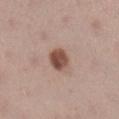Part of a total-body skin-imaging series; this lesion was reviewed on a skin check and was not flagged for biopsy. A close-up tile cropped from a whole-body skin photograph, about 15 mm across. The lesion-visualizer software estimated an average lesion color of about L≈49 a*≈20 b*≈25 (CIELAB), about 15 CIELAB-L* units darker than the surrounding skin, and a normalized lesion–skin contrast near 10.5. It also reported a color-variation rating of about 4/10 and peripheral color asymmetry of about 1.5. And it measured a nevus-likeness score of about 100/100 and lesion-presence confidence of about 100/100. A female patient aged 38–42. This is a white-light tile. On the leg.